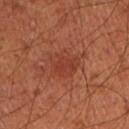| key | value |
|---|---|
| notes | catalogued during a skin exam; not biopsied |
| illumination | cross-polarized |
| site | the left upper arm |
| patient | male, aged around 50 |
| lesion diameter | ~3 mm (longest diameter) |
| TBP lesion metrics | an area of roughly 8 mm², a shape eccentricity near 0.5, and two-axis asymmetry of about 0.15; a nevus-likeness score of about 20/100 |
| imaging modality | ~15 mm crop, total-body skin-cancer survey |